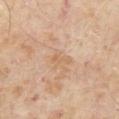Clinical impression: The lesion was photographed on a routine skin check and not biopsied; there is no pathology result. Clinical summary: Captured under cross-polarized illumination. Longest diameter approximately 2.5 mm. A male subject roughly 65 years of age. A close-up tile cropped from a whole-body skin photograph, about 15 mm across.